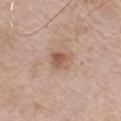<tbp_lesion>
  <biopsy_status>not biopsied; imaged during a skin examination</biopsy_status>
  <site>chest</site>
  <lesion_size>
    <long_diameter_mm_approx>3.0</long_diameter_mm_approx>
  </lesion_size>
  <patient>
    <sex>male</sex>
    <age_approx>65</age_approx>
  </patient>
  <lighting>white-light</lighting>
  <automated_metrics>
    <area_mm2_approx>5.5</area_mm2_approx>
    <eccentricity>0.45</eccentricity>
    <shape_asymmetry>0.2</shape_asymmetry>
    <color_variation_0_10>4.5</color_variation_0_10>
    <peripheral_color_asymmetry>1.5</peripheral_color_asymmetry>
  </automated_metrics>
  <image>
    <source>total-body photography crop</source>
    <field_of_view_mm>15</field_of_view_mm>
  </image>
</tbp_lesion>